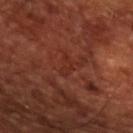<lesion>
<patient>
  <sex>male</sex>
  <age_approx>65</age_approx>
</patient>
<lighting>cross-polarized</lighting>
<image>
  <source>total-body photography crop</source>
  <field_of_view_mm>15</field_of_view_mm>
</image>
<lesion_size>
  <long_diameter_mm_approx>3.0</long_diameter_mm_approx>
</lesion_size>
</lesion>